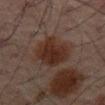notes: catalogued during a skin exam; not biopsied
body site: the lower back
image source: ~15 mm crop, total-body skin-cancer survey
size: about 5 mm
patient: male, aged 63–67